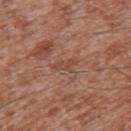notes: total-body-photography surveillance lesion; no biopsy | image source: ~15 mm crop, total-body skin-cancer survey | size: ≈3 mm | TBP lesion metrics: an area of roughly 3 mm², an outline eccentricity of about 0.9 (0 = round, 1 = elongated), and a symmetry-axis asymmetry near 0.4; a mean CIELAB color near L≈47 a*≈22 b*≈29, about 7 CIELAB-L* units darker than the surrounding skin, and a normalized border contrast of about 5; border irregularity of about 4.5 on a 0–10 scale and radial color variation of about 0; a classifier nevus-likeness of about 0/100 and a lesion-detection confidence of about 95/100 | subject: male, roughly 45 years of age | site: the left upper arm.